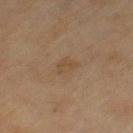| field | value |
|---|---|
| workup | total-body-photography surveillance lesion; no biopsy |